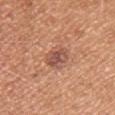biopsy status: no biopsy performed (imaged during a skin exam) | subject: male, about 30 years old | image source: ~15 mm crop, total-body skin-cancer survey | site: the left upper arm | tile lighting: white-light illumination | automated lesion analysis: a footprint of about 7 mm², an outline eccentricity of about 0.8 (0 = round, 1 = elongated), and two-axis asymmetry of about 0.15; a border-irregularity index near 1.5/10, a within-lesion color-variation index near 4.5/10, and a peripheral color-asymmetry measure near 1.5.